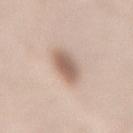Clinical impression:
Captured during whole-body skin photography for melanoma surveillance; the lesion was not biopsied.